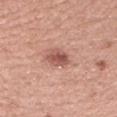Part of a total-body skin-imaging series; this lesion was reviewed on a skin check and was not flagged for biopsy. Captured under white-light illumination. Longest diameter approximately 3 mm. A male subject in their 40s. Located on the left upper arm. This image is a 15 mm lesion crop taken from a total-body photograph.